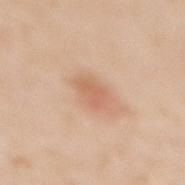{"biopsy_status": "not biopsied; imaged during a skin examination", "lighting": "white-light", "automated_metrics": {"vs_skin_darker_L": 8.0, "vs_skin_contrast_norm": 5.5, "lesion_detection_confidence_0_100": 100}, "site": "back", "patient": {"sex": "female", "age_approx": 40}, "image": {"source": "total-body photography crop", "field_of_view_mm": 15}, "lesion_size": {"long_diameter_mm_approx": 3.0}}